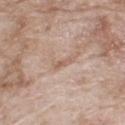Recorded during total-body skin imaging; not selected for excision or biopsy. About 3 mm across. A male patient, roughly 65 years of age. Cropped from a total-body skin-imaging series; the visible field is about 15 mm. The total-body-photography lesion software estimated a footprint of about 3 mm². The analysis additionally found an average lesion color of about L≈60 a*≈17 b*≈28 (CIELAB), roughly 7 lightness units darker than nearby skin, and a normalized border contrast of about 5.5. The analysis additionally found an automated nevus-likeness rating near 0 out of 100 and lesion-presence confidence of about 70/100. Imaged with white-light lighting. Located on the upper back.